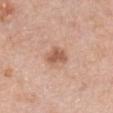Captured during whole-body skin photography for melanoma surveillance; the lesion was not biopsied.